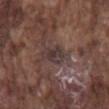| field | value |
|---|---|
| body site | the mid back |
| subject | male, in their mid-70s |
| acquisition | total-body-photography crop, ~15 mm field of view |